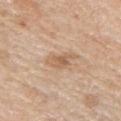Part of a total-body skin-imaging series; this lesion was reviewed on a skin check and was not flagged for biopsy. A region of skin cropped from a whole-body photographic capture, roughly 15 mm wide. The lesion is on the right upper arm. Approximately 3.5 mm at its widest. The patient is a male roughly 60 years of age.